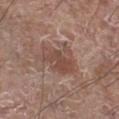Q: Is there a histopathology result?
A: imaged on a skin check; not biopsied
Q: What is the lesion's diameter?
A: about 4.5 mm
Q: Who is the patient?
A: male, aged 58–62
Q: How was this image acquired?
A: 15 mm crop, total-body photography
Q: Illumination type?
A: white-light
Q: Where on the body is the lesion?
A: the leg